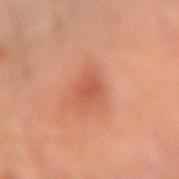Clinical impression: Imaged during a routine full-body skin examination; the lesion was not biopsied and no histopathology is available. Clinical summary: Approximately 2.5 mm at its widest. A male subject in their 70s. The tile uses cross-polarized illumination. A lesion tile, about 15 mm wide, cut from a 3D total-body photograph. Automated image analysis of the tile measured two-axis asymmetry of about 0.2. And it measured a lesion–skin lightness drop of about 8 and a normalized lesion–skin contrast near 5.5. The software also gave a border-irregularity rating of about 1.5/10, internal color variation of about 2.5 on a 0–10 scale, and peripheral color asymmetry of about 1. It also reported a classifier nevus-likeness of about 55/100 and lesion-presence confidence of about 100/100. From the arm.